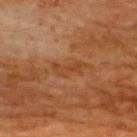A male subject, aged 63–67. Located on the upper back. Longest diameter approximately 4 mm. A region of skin cropped from a whole-body photographic capture, roughly 15 mm wide. Captured under cross-polarized illumination. Automated image analysis of the tile measured a lesion color around L≈43 a*≈24 b*≈37 in CIELAB, about 6 CIELAB-L* units darker than the surrounding skin, and a normalized lesion–skin contrast near 5. The software also gave a border-irregularity index near 5/10, a within-lesion color-variation index near 4/10, and radial color variation of about 1.5.